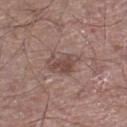The lesion was photographed on a routine skin check and not biopsied; there is no pathology result. A lesion tile, about 15 mm wide, cut from a 3D total-body photograph. Measured at roughly 4 mm in maximum diameter. Captured under white-light illumination. The lesion is on the right lower leg. A male subject about 55 years old. The total-body-photography lesion software estimated a lesion color around L≈47 a*≈18 b*≈21 in CIELAB, a lesion–skin lightness drop of about 9, and a normalized border contrast of about 7.